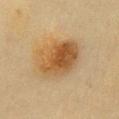follow-up — no biopsy performed (imaged during a skin exam); body site — the chest; acquisition — ~15 mm tile from a whole-body skin photo; lesion size — ~6 mm (longest diameter); patient — female, in their 60s; illumination — cross-polarized illumination.